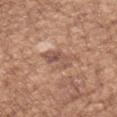Q: Automated lesion metrics?
A: an average lesion color of about L≈53 a*≈20 b*≈27 (CIELAB), a lesion–skin lightness drop of about 9, and a normalized lesion–skin contrast near 6.5; a classifier nevus-likeness of about 0/100 and lesion-presence confidence of about 90/100
Q: What are the patient's age and sex?
A: female, in their mid- to late 70s
Q: Lesion location?
A: the left upper arm
Q: How large is the lesion?
A: ≈3.5 mm
Q: What is the imaging modality?
A: total-body-photography crop, ~15 mm field of view